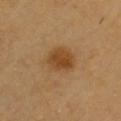No biopsy was performed on this lesion — it was imaged during a full skin examination and was not determined to be concerning.
The tile uses cross-polarized illumination.
Cropped from a whole-body photographic skin survey; the tile spans about 15 mm.
A male patient, aged 58 to 62.
On the front of the torso.